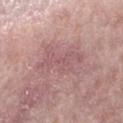Assessment:
The lesion was tiled from a total-body skin photograph and was not biopsied.
Background:
This image is a 15 mm lesion crop taken from a total-body photograph. Approximately 6 mm at its widest. Captured under white-light illumination. On the right forearm. The patient is a female in their 70s.